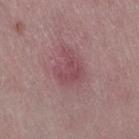Impression: The lesion was tiled from a total-body skin photograph and was not biopsied. Clinical summary: A 15 mm crop from a total-body photograph taken for skin-cancer surveillance. From the right thigh. A female patient in their mid- to late 40s.